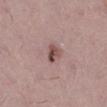Q: Was a biopsy performed?
A: total-body-photography surveillance lesion; no biopsy